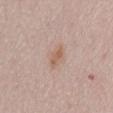{"lighting": "white-light", "site": "abdomen", "lesion_size": {"long_diameter_mm_approx": 3.0}, "image": {"source": "total-body photography crop", "field_of_view_mm": 15}, "patient": {"sex": "female", "age_approx": 50}, "automated_metrics": {"area_mm2_approx": 4.0, "shape_asymmetry": 0.25, "cielab_L": 60, "cielab_a": 18, "cielab_b": 28, "vs_skin_darker_L": 8.0, "vs_skin_contrast_norm": 6.5, "nevus_likeness_0_100": 35, "lesion_detection_confidence_0_100": 100}}